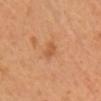The lesion-visualizer software estimated a shape eccentricity near 0.8 and two-axis asymmetry of about 0.25. The software also gave a classifier nevus-likeness of about 0/100. The tile uses cross-polarized illumination. A female subject. Located on the chest. Measured at roughly 2.5 mm in maximum diameter. A close-up tile cropped from a whole-body skin photograph, about 15 mm across.